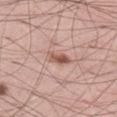tile lighting = white-light; site = the leg; subject = male, in their mid-50s; imaging modality = 15 mm crop, total-body photography; image-analysis metrics = a classifier nevus-likeness of about 85/100 and a lesion-detection confidence of about 100/100.